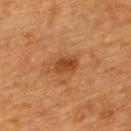Clinical impression:
Part of a total-body skin-imaging series; this lesion was reviewed on a skin check and was not flagged for biopsy.
Image and clinical context:
The total-body-photography lesion software estimated an area of roughly 5 mm², an outline eccentricity of about 0.75 (0 = round, 1 = elongated), and two-axis asymmetry of about 0.2. The analysis additionally found a border-irregularity rating of about 2/10, a color-variation rating of about 3/10, and peripheral color asymmetry of about 1. The software also gave a classifier nevus-likeness of about 80/100 and a detector confidence of about 100 out of 100 that the crop contains a lesion. The patient is a female about 55 years old. The lesion is on the upper back. Cropped from a whole-body photographic skin survey; the tile spans about 15 mm. The tile uses cross-polarized illumination. The lesion's longest dimension is about 2.5 mm.